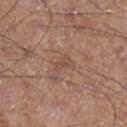The lesion was tiled from a total-body skin photograph and was not biopsied.
The lesion's longest dimension is about 2.5 mm.
A male subject, about 60 years old.
A 15 mm close-up extracted from a 3D total-body photography capture.
From the right lower leg.
The total-body-photography lesion software estimated an area of roughly 2.5 mm², a shape eccentricity near 0.9, and a symmetry-axis asymmetry near 0.4. The software also gave a mean CIELAB color near L≈49 a*≈20 b*≈26, about 6 CIELAB-L* units darker than the surrounding skin, and a lesion-to-skin contrast of about 5 (normalized; higher = more distinct). The analysis additionally found a border-irregularity index near 4/10 and radial color variation of about 0. It also reported an automated nevus-likeness rating near 0 out of 100 and lesion-presence confidence of about 95/100.
Imaged with white-light lighting.